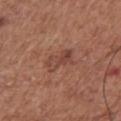No biopsy was performed on this lesion — it was imaged during a full skin examination and was not determined to be concerning. Measured at roughly 3.5 mm in maximum diameter. A male patient, aged approximately 75. A 15 mm close-up tile from a total-body photography series done for melanoma screening. An algorithmic analysis of the crop reported a lesion area of about 6 mm², an outline eccentricity of about 0.85 (0 = round, 1 = elongated), and a shape-asymmetry score of about 0.35 (0 = symmetric). The software also gave a lesion color around L≈44 a*≈23 b*≈27 in CIELAB, roughly 8 lightness units darker than nearby skin, and a normalized border contrast of about 6. It also reported a nevus-likeness score of about 0/100. Located on the chest.